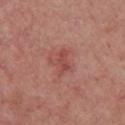Imaged during a routine full-body skin examination; the lesion was not biopsied and no histopathology is available. The lesion is located on the chest. A close-up tile cropped from a whole-body skin photograph, about 15 mm across. The lesion-visualizer software estimated an area of roughly 5 mm² and a shape eccentricity near 0.75. The software also gave a mean CIELAB color near L≈48 a*≈28 b*≈26, roughly 8 lightness units darker than nearby skin, and a lesion-to-skin contrast of about 6 (normalized; higher = more distinct). About 3 mm across. A male subject aged around 60. Captured under white-light illumination.